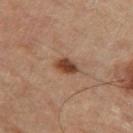workup: total-body-photography surveillance lesion; no biopsy | subject: male, aged 83 or over | lighting: cross-polarized illumination | diameter: about 2.5 mm | image-analysis metrics: a border-irregularity rating of about 2/10, a within-lesion color-variation index near 2.5/10, and a peripheral color-asymmetry measure near 1; a detector confidence of about 100 out of 100 that the crop contains a lesion | image source: ~15 mm tile from a whole-body skin photo | body site: the left thigh.